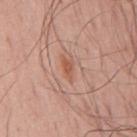  site: mid back
  lighting: white-light
  image:
    source: total-body photography crop
    field_of_view_mm: 15
  patient:
    sex: male
    age_approx: 65
  automated_metrics:
    eccentricity: 0.9
    shape_asymmetry: 0.35
    nevus_likeness_0_100: 0
  lesion_size:
    long_diameter_mm_approx: 3.0